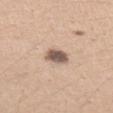Part of a total-body skin-imaging series; this lesion was reviewed on a skin check and was not flagged for biopsy. On the arm. Measured at roughly 3 mm in maximum diameter. The patient is a female approximately 25 years of age. A 15 mm close-up tile from a total-body photography series done for melanoma screening.